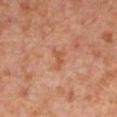{
  "biopsy_status": "not biopsied; imaged during a skin examination",
  "lighting": "cross-polarized",
  "image": {
    "source": "total-body photography crop",
    "field_of_view_mm": 15
  },
  "site": "left lower leg",
  "patient": {
    "sex": "male",
    "age_approx": 30
  },
  "automated_metrics": {
    "area_mm2_approx": 3.0,
    "shape_asymmetry": 0.55,
    "vs_skin_darker_L": 7.0,
    "vs_skin_contrast_norm": 6.5,
    "nevus_likeness_0_100": 0
  },
  "lesion_size": {
    "long_diameter_mm_approx": 2.5
  }
}